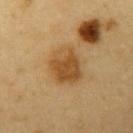Imaged during a routine full-body skin examination; the lesion was not biopsied and no histopathology is available.
This image is a 15 mm lesion crop taken from a total-body photograph.
From the left upper arm.
A male patient about 60 years old.